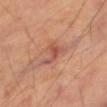imaging modality: ~15 mm tile from a whole-body skin photo; site: the right thigh; image-analysis metrics: an area of roughly 6 mm², an outline eccentricity of about 0.85 (0 = round, 1 = elongated), and a symmetry-axis asymmetry near 0.6; lesion diameter: ≈4 mm; illumination: cross-polarized; subject: male, aged 68 to 72.